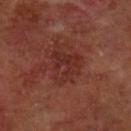Captured during whole-body skin photography for melanoma surveillance; the lesion was not biopsied.
Longest diameter approximately 4.5 mm.
A male subject roughly 70 years of age.
The lesion is on the arm.
A roughly 15 mm field-of-view crop from a total-body skin photograph.